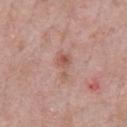Recorded during total-body skin imaging; not selected for excision or biopsy. From the chest. The tile uses white-light illumination. A male subject, aged around 55. About 3.5 mm across. This image is a 15 mm lesion crop taken from a total-body photograph.